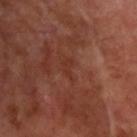Recorded during total-body skin imaging; not selected for excision or biopsy.
The lesion is on the left upper arm.
A lesion tile, about 15 mm wide, cut from a 3D total-body photograph.
A male patient in their mid-60s.